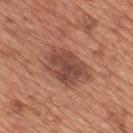Captured under white-light illumination. On the mid back. A male subject, roughly 65 years of age. A lesion tile, about 15 mm wide, cut from a 3D total-body photograph. Longest diameter approximately 5.5 mm.